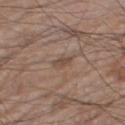Clinical impression:
Part of a total-body skin-imaging series; this lesion was reviewed on a skin check and was not flagged for biopsy.
Image and clinical context:
Located on the right thigh. A lesion tile, about 15 mm wide, cut from a 3D total-body photograph. The patient is a male aged around 70.